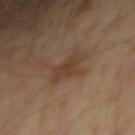Notes:
- follow-up · imaged on a skin check; not biopsied
- acquisition · 15 mm crop, total-body photography
- location · the right upper arm
- patient · male, aged 53 to 57
- illumination · cross-polarized